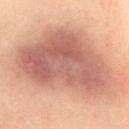biopsy_status: not biopsied; imaged during a skin examination
image:
  source: total-body photography crop
  field_of_view_mm: 15
automated_metrics:
  area_mm2_approx: 80.0
  eccentricity: 0.7
  shape_asymmetry: 0.3
lesion_size:
  long_diameter_mm_approx: 13.0
site: abdomen
patient:
  sex: female
  age_approx: 40
lighting: cross-polarized